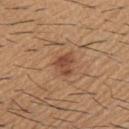Captured during whole-body skin photography for melanoma surveillance; the lesion was not biopsied.
Cropped from a total-body skin-imaging series; the visible field is about 15 mm.
Imaged with white-light lighting.
A male patient in their 60s.
The lesion is on the upper back.
The total-body-photography lesion software estimated a footprint of about 4.5 mm², a shape eccentricity near 0.6, and a symmetry-axis asymmetry near 0.4. It also reported a nevus-likeness score of about 65/100 and lesion-presence confidence of about 100/100.